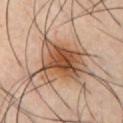The lesion was photographed on a routine skin check and not biopsied; there is no pathology result.
A lesion tile, about 15 mm wide, cut from a 3D total-body photograph.
A male patient, aged around 40.
About 6.5 mm across.
This is a cross-polarized tile.
From the chest.